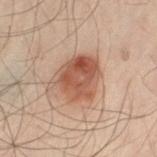Context: This is a cross-polarized tile. The lesion is located on the left thigh. A 15 mm close-up extracted from a 3D total-body photography capture. The lesion-visualizer software estimated an average lesion color of about L≈43 a*≈19 b*≈26 (CIELAB), about 11 CIELAB-L* units darker than the surrounding skin, and a lesion-to-skin contrast of about 8.5 (normalized; higher = more distinct). The analysis additionally found a border-irregularity index near 2.5/10, internal color variation of about 5.5 on a 0–10 scale, and radial color variation of about 2. The software also gave a nevus-likeness score of about 95/100 and lesion-presence confidence of about 100/100. A male subject about 50 years old.